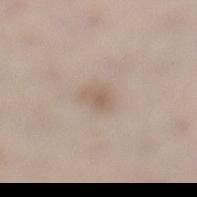The lesion is on the leg. The recorded lesion diameter is about 2.5 mm. The tile uses white-light illumination. A roughly 15 mm field-of-view crop from a total-body skin photograph. The patient is a female roughly 40 years of age. An algorithmic analysis of the crop reported an area of roughly 3.5 mm² and a shape eccentricity near 0.75. The analysis additionally found a mean CIELAB color near L≈59 a*≈14 b*≈26, about 8 CIELAB-L* units darker than the surrounding skin, and a normalized lesion–skin contrast near 6. The analysis additionally found a color-variation rating of about 1.5/10 and radial color variation of about 0.5. It also reported an automated nevus-likeness rating near 65 out of 100 and lesion-presence confidence of about 100/100.